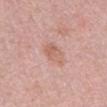Case summary:
- follow-up · catalogued during a skin exam; not biopsied
- patient · male, in their 50s
- anatomic site · the chest
- imaging modality · ~15 mm crop, total-body skin-cancer survey
- lesion diameter · ~3.5 mm (longest diameter)
- lighting · white-light illumination